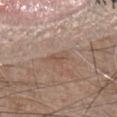This lesion was catalogued during total-body skin photography and was not selected for biopsy. A male subject, aged around 45. Located on the front of the torso. A close-up tile cropped from a whole-body skin photograph, about 15 mm across.